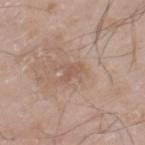Q: Was a biopsy performed?
A: catalogued during a skin exam; not biopsied
Q: Lesion location?
A: the leg
Q: What lighting was used for the tile?
A: white-light illumination
Q: What is the imaging modality?
A: ~15 mm crop, total-body skin-cancer survey
Q: Automated lesion metrics?
A: about 6 CIELAB-L* units darker than the surrounding skin and a normalized border contrast of about 5; a detector confidence of about 100 out of 100 that the crop contains a lesion
Q: Patient demographics?
A: male, approximately 60 years of age
Q: How large is the lesion?
A: ≈2.5 mm